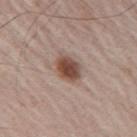Q: Patient demographics?
A: male, about 65 years old
Q: What is the imaging modality?
A: total-body-photography crop, ~15 mm field of view
Q: What is the lesion's diameter?
A: about 3.5 mm
Q: What did automated image analysis measure?
A: a lesion color around L≈48 a*≈19 b*≈25 in CIELAB, roughly 14 lightness units darker than nearby skin, and a normalized lesion–skin contrast near 10.5; internal color variation of about 5 on a 0–10 scale and radial color variation of about 1.5; a detector confidence of about 100 out of 100 that the crop contains a lesion
Q: What is the anatomic site?
A: the right upper arm
Q: What lighting was used for the tile?
A: white-light